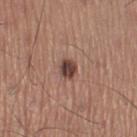Notes:
• notes — no biopsy performed (imaged during a skin exam)
• subject — male, approximately 60 years of age
• lighting — white-light
• body site — the right thigh
• automated lesion analysis — an average lesion color of about L≈42 a*≈20 b*≈23 (CIELAB)
• acquisition — 15 mm crop, total-body photography